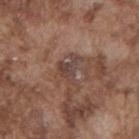biopsy_status: not biopsied; imaged during a skin examination
lesion_size:
  long_diameter_mm_approx: 3.5
image:
  source: total-body photography crop
  field_of_view_mm: 15
patient:
  sex: male
  age_approx: 75
site: back
lighting: white-light